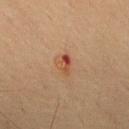Q: Is there a histopathology result?
A: no biopsy performed (imaged during a skin exam)
Q: What did automated image analysis measure?
A: a footprint of about 4.5 mm² and a shape-asymmetry score of about 0.25 (0 = symmetric); a border-irregularity index near 2.5/10 and internal color variation of about 10 on a 0–10 scale
Q: What lighting was used for the tile?
A: cross-polarized
Q: What is the anatomic site?
A: the upper back
Q: Who is the patient?
A: male, aged 68–72
Q: What is the imaging modality?
A: ~15 mm tile from a whole-body skin photo